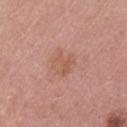Q: Is there a histopathology result?
A: imaged on a skin check; not biopsied
Q: Lesion location?
A: the left upper arm
Q: What did automated image analysis measure?
A: a footprint of about 7 mm², an outline eccentricity of about 0.55 (0 = round, 1 = elongated), and a symmetry-axis asymmetry near 0.25; an average lesion color of about L≈57 a*≈23 b*≈29 (CIELAB), roughly 6 lightness units darker than nearby skin, and a lesion-to-skin contrast of about 5 (normalized; higher = more distinct); a border-irregularity index near 3.5/10, a color-variation rating of about 2.5/10, and a peripheral color-asymmetry measure near 1; a nevus-likeness score of about 5/100
Q: Lesion size?
A: about 3 mm
Q: How was the tile lit?
A: white-light
Q: What is the imaging modality?
A: 15 mm crop, total-body photography
Q: Patient demographics?
A: female, in their mid- to late 40s